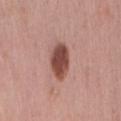The lesion was tiled from a total-body skin photograph and was not biopsied.
Cropped from a total-body skin-imaging series; the visible field is about 15 mm.
Longest diameter approximately 4 mm.
The tile uses white-light illumination.
The lesion is on the back.
A female subject, in their 40s.